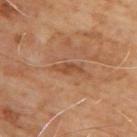Q: What is the lesion's diameter?
A: about 3.5 mm
Q: What did automated image analysis measure?
A: a footprint of about 3.5 mm², a shape eccentricity near 0.95, and a symmetry-axis asymmetry near 0.35; border irregularity of about 4 on a 0–10 scale, internal color variation of about 1 on a 0–10 scale, and a peripheral color-asymmetry measure near 0.5
Q: Where on the body is the lesion?
A: the upper back
Q: Patient demographics?
A: male, aged 63–67
Q: What is the imaging modality?
A: ~15 mm tile from a whole-body skin photo